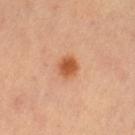biopsy status: no biopsy performed (imaged during a skin exam); image source: 15 mm crop, total-body photography; anatomic site: the leg; subject: female, aged 48 to 52.